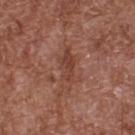Case summary:
- follow-up: catalogued during a skin exam; not biopsied
- image source: total-body-photography crop, ~15 mm field of view
- lesion size: about 5 mm
- tile lighting: white-light illumination
- site: the back
- patient: male, aged approximately 75